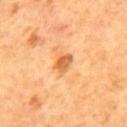anatomic site: the mid back | subject: male, aged around 55 | image-analysis metrics: a lesion color around L≈59 a*≈26 b*≈43 in CIELAB and a lesion-to-skin contrast of about 7.5 (normalized; higher = more distinct); a border-irregularity index near 2.5/10, internal color variation of about 3 on a 0–10 scale, and radial color variation of about 1; an automated nevus-likeness rating near 45 out of 100 and lesion-presence confidence of about 100/100 | size: about 2.5 mm | lighting: cross-polarized | image: total-body-photography crop, ~15 mm field of view.